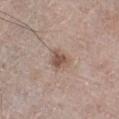| field | value |
|---|---|
| follow-up | catalogued during a skin exam; not biopsied |
| anatomic site | the leg |
| lesion diameter | about 2.5 mm |
| patient | male, roughly 70 years of age |
| automated lesion analysis | a footprint of about 4.5 mm² and a shape eccentricity near 0.55; a mean CIELAB color near L≈52 a*≈17 b*≈25 and a normalized border contrast of about 7.5; a color-variation rating of about 3.5/10 and radial color variation of about 1 |
| lighting | white-light illumination |
| imaging modality | 15 mm crop, total-body photography |